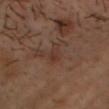Findings:
- follow-up · catalogued during a skin exam; not biopsied
- diameter · ~3 mm (longest diameter)
- site · the head or neck
- acquisition · ~15 mm crop, total-body skin-cancer survey
- lighting · cross-polarized illumination
- patient · male, in their 50s